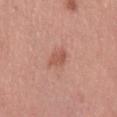Q: Was a biopsy performed?
A: imaged on a skin check; not biopsied
Q: Patient demographics?
A: female, aged 63 to 67
Q: How was this image acquired?
A: ~15 mm tile from a whole-body skin photo
Q: What is the anatomic site?
A: the right thigh
Q: Lesion size?
A: ≈2.5 mm
Q: What lighting was used for the tile?
A: white-light illumination
Q: What did automated image analysis measure?
A: a lesion area of about 4 mm², an eccentricity of roughly 0.65, and a shape-asymmetry score of about 0.2 (0 = symmetric); an average lesion color of about L≈55 a*≈25 b*≈29 (CIELAB) and a lesion-to-skin contrast of about 6 (normalized; higher = more distinct)